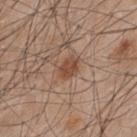Impression: The lesion was photographed on a routine skin check and not biopsied; there is no pathology result. Clinical summary: This is a white-light tile. The recorded lesion diameter is about 3 mm. The subject is a male approximately 45 years of age. A close-up tile cropped from a whole-body skin photograph, about 15 mm across. The lesion is located on the upper back.